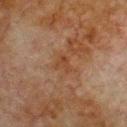Part of a total-body skin-imaging series; this lesion was reviewed on a skin check and was not flagged for biopsy. This is a cross-polarized tile. Located on the chest. A male subject, aged 78 to 82. An algorithmic analysis of the crop reported a footprint of about 3.5 mm², a shape eccentricity near 0.7, and a symmetry-axis asymmetry near 0.5. The analysis additionally found an average lesion color of about L≈34 a*≈18 b*≈27 (CIELAB) and a lesion–skin lightness drop of about 5. The lesion's longest dimension is about 2.5 mm. A 15 mm close-up extracted from a 3D total-body photography capture.